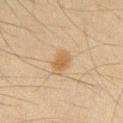Part of a total-body skin-imaging series; this lesion was reviewed on a skin check and was not flagged for biopsy. Measured at roughly 2.5 mm in maximum diameter. A male subject, about 35 years old. This image is a 15 mm lesion crop taken from a total-body photograph. The tile uses cross-polarized illumination. An algorithmic analysis of the crop reported a footprint of about 4.5 mm², an outline eccentricity of about 0.6 (0 = round, 1 = elongated), and a shape-asymmetry score of about 0.25 (0 = symmetric). It also reported a border-irregularity index near 2/10, a color-variation rating of about 2/10, and radial color variation of about 0.5. It also reported an automated nevus-likeness rating near 85 out of 100 and a lesion-detection confidence of about 100/100. On the chest.